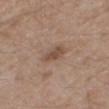Recorded during total-body skin imaging; not selected for excision or biopsy.
A male subject, about 65 years old.
Approximately 3.5 mm at its widest.
The tile uses white-light illumination.
A roughly 15 mm field-of-view crop from a total-body skin photograph.
The lesion-visualizer software estimated border irregularity of about 2.5 on a 0–10 scale, a within-lesion color-variation index near 1.5/10, and a peripheral color-asymmetry measure near 0.5. The analysis additionally found an automated nevus-likeness rating near 5 out of 100 and a lesion-detection confidence of about 100/100.
On the right thigh.